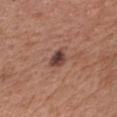Findings:
• follow-up: total-body-photography surveillance lesion; no biopsy
• location: the chest
• patient: male, aged approximately 55
• acquisition: ~15 mm tile from a whole-body skin photo